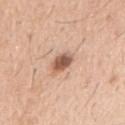<case>
  <biopsy_status>not biopsied; imaged during a skin examination</biopsy_status>
  <site>arm</site>
  <automated_metrics>
    <area_mm2_approx>5.0</area_mm2_approx>
    <eccentricity>0.5</eccentricity>
    <border_irregularity_0_10>2.0</border_irregularity_0_10>
    <color_variation_0_10>4.0</color_variation_0_10>
    <peripheral_color_asymmetry>1.5</peripheral_color_asymmetry>
    <nevus_likeness_0_100>95</nevus_likeness_0_100>
    <lesion_detection_confidence_0_100>100</lesion_detection_confidence_0_100>
  </automated_metrics>
  <lighting>white-light</lighting>
  <image>
    <source>total-body photography crop</source>
    <field_of_view_mm>15</field_of_view_mm>
  </image>
  <patient>
    <sex>male</sex>
    <age_approx>40</age_approx>
  </patient>
</case>